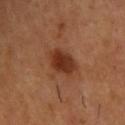Captured during whole-body skin photography for melanoma surveillance; the lesion was not biopsied.
A male subject, aged 53–57.
The tile uses cross-polarized illumination.
Cropped from a whole-body photographic skin survey; the tile spans about 15 mm.
Automated tile analysis of the lesion measured an average lesion color of about L≈33 a*≈24 b*≈31 (CIELAB). It also reported an automated nevus-likeness rating near 90 out of 100.
The lesion's longest dimension is about 4 mm.
The lesion is located on the upper back.